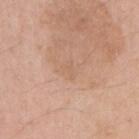Background: Located on the right upper arm. The lesion's longest dimension is about 2 mm. The patient is a female approximately 55 years of age. A close-up tile cropped from a whole-body skin photograph, about 15 mm across. The tile uses white-light illumination.